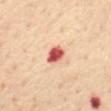Findings:
- follow-up: total-body-photography surveillance lesion; no biopsy
- body site: the mid back
- lesion size: about 3 mm
- lighting: cross-polarized illumination
- patient: female, aged around 70
- image source: total-body-photography crop, ~15 mm field of view